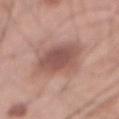Clinical impression:
Captured during whole-body skin photography for melanoma surveillance; the lesion was not biopsied.
Clinical summary:
A 15 mm crop from a total-body photograph taken for skin-cancer surveillance. From the front of the torso. The subject is a male about 70 years old.